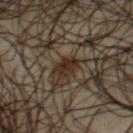| key | value |
|---|---|
| notes | catalogued during a skin exam; not biopsied |
| patient | male, aged approximately 65 |
| lighting | cross-polarized |
| site | the chest |
| acquisition | ~15 mm tile from a whole-body skin photo |
| size | ~2.5 mm (longest diameter) |